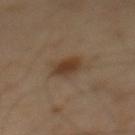| key | value |
|---|---|
| workup | imaged on a skin check; not biopsied |
| image source | ~15 mm tile from a whole-body skin photo |
| patient | male, about 40 years old |
| location | the mid back |
| automated metrics | a mean CIELAB color near L≈38 a*≈15 b*≈29, roughly 9 lightness units darker than nearby skin, and a normalized border contrast of about 8.5; a border-irregularity index near 2/10 and radial color variation of about 1.5 |
| lesion diameter | ~3 mm (longest diameter) |